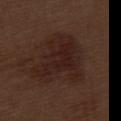<record>
<biopsy_status>not biopsied; imaged during a skin examination</biopsy_status>
<site>left thigh</site>
<image>
  <source>total-body photography crop</source>
  <field_of_view_mm>15</field_of_view_mm>
</image>
<patient>
  <sex>male</sex>
  <age_approx>70</age_approx>
</patient>
</record>